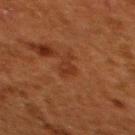• notes · total-body-photography surveillance lesion; no biopsy
• image · total-body-photography crop, ~15 mm field of view
• site · the back
• subject · female, aged approximately 50